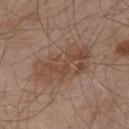Captured during whole-body skin photography for melanoma surveillance; the lesion was not biopsied. A male subject, aged 53–57. On the upper back. Captured under white-light illumination. Measured at roughly 7.5 mm in maximum diameter. Cropped from a total-body skin-imaging series; the visible field is about 15 mm.